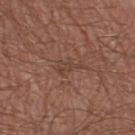{
  "lesion_size": {
    "long_diameter_mm_approx": 3.0
  },
  "image": {
    "source": "total-body photography crop",
    "field_of_view_mm": 15
  },
  "lighting": "white-light",
  "patient": {
    "sex": "male",
    "age_approx": 65
  },
  "site": "leg",
  "automated_metrics": {
    "area_mm2_approx": 2.5,
    "eccentricity": 0.9,
    "shape_asymmetry": 0.5,
    "cielab_L": 41,
    "cielab_a": 18,
    "cielab_b": 25
  }
}